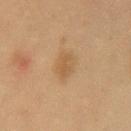* follow-up — no biopsy performed (imaged during a skin exam)
* site — the chest
* subject — female, aged around 60
* lighting — cross-polarized illumination
* imaging modality — total-body-photography crop, ~15 mm field of view